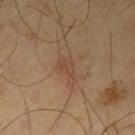Assessment: Recorded during total-body skin imaging; not selected for excision or biopsy. Image and clinical context: Imaged with cross-polarized lighting. Cropped from a total-body skin-imaging series; the visible field is about 15 mm. A male subject, aged 53 to 57. Located on the left thigh. Approximately 2 mm at its widest.